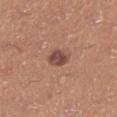notes: imaged on a skin check; not biopsied | size: ≈3 mm | site: the left lower leg | lighting: white-light | patient: male, aged around 40 | image: total-body-photography crop, ~15 mm field of view | automated metrics: a shape-asymmetry score of about 0.2 (0 = symmetric); a border-irregularity index near 2/10, internal color variation of about 2.5 on a 0–10 scale, and peripheral color asymmetry of about 1; a classifier nevus-likeness of about 60/100 and a detector confidence of about 100 out of 100 that the crop contains a lesion.